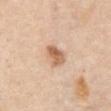workup=catalogued during a skin exam; not biopsied
automated metrics=a mean CIELAB color near L≈63 a*≈20 b*≈34, about 13 CIELAB-L* units darker than the surrounding skin, and a normalized border contrast of about 8
subject=female, about 65 years old
anatomic site=the abdomen
acquisition=total-body-photography crop, ~15 mm field of view